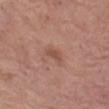The lesion was tiled from a total-body skin photograph and was not biopsied. Captured under white-light illumination. From the left thigh. Cropped from a total-body skin-imaging series; the visible field is about 15 mm. A female subject approximately 65 years of age. An algorithmic analysis of the crop reported an area of roughly 3 mm², an outline eccentricity of about 0.85 (0 = round, 1 = elongated), and two-axis asymmetry of about 0.3. The software also gave an average lesion color of about L≈51 a*≈23 b*≈27 (CIELAB), roughly 8 lightness units darker than nearby skin, and a lesion-to-skin contrast of about 6 (normalized; higher = more distinct). The software also gave a border-irregularity rating of about 3/10 and a within-lesion color-variation index near 1/10.